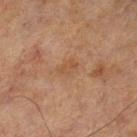The lesion was photographed on a routine skin check and not biopsied; there is no pathology result. Longest diameter approximately 2.5 mm. A 15 mm close-up tile from a total-body photography series done for melanoma screening. A male subject, roughly 70 years of age. On the left lower leg.